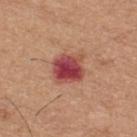biopsy status: no biopsy performed (imaged during a skin exam); lesion size: ~3.5 mm (longest diameter); subject: male, aged 58 to 62; body site: the upper back; acquisition: ~15 mm crop, total-body skin-cancer survey.